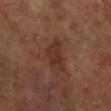The lesion is on the right lower leg. A subject aged 63–67. A roughly 15 mm field-of-view crop from a total-body skin photograph.